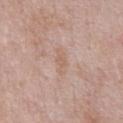<tbp_lesion>
  <biopsy_status>not biopsied; imaged during a skin examination</biopsy_status>
  <site>chest</site>
  <image>
    <source>total-body photography crop</source>
    <field_of_view_mm>15</field_of_view_mm>
  </image>
  <patient>
    <sex>male</sex>
    <age_approx>55</age_approx>
  </patient>
</tbp_lesion>